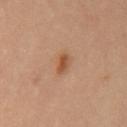From the front of the torso.
A roughly 15 mm field-of-view crop from a total-body skin photograph.
Measured at roughly 3 mm in maximum diameter.
A male patient, in their mid- to late 60s.
Automated tile analysis of the lesion measured a lesion area of about 3 mm², an eccentricity of roughly 0.9, and two-axis asymmetry of about 0.3. The software also gave an average lesion color of about L≈51 a*≈23 b*≈36 (CIELAB), roughly 10 lightness units darker than nearby skin, and a normalized lesion–skin contrast near 8. And it measured a border-irregularity rating of about 3/10, internal color variation of about 1 on a 0–10 scale, and peripheral color asymmetry of about 0.5. The software also gave a classifier nevus-likeness of about 90/100 and a lesion-detection confidence of about 100/100.